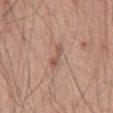biopsy_status: not biopsied; imaged during a skin examination
image:
  source: total-body photography crop
  field_of_view_mm: 15
site: chest
lesion_size:
  long_diameter_mm_approx: 2.5
patient:
  sex: male
  age_approx: 55